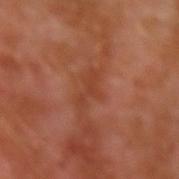Q: Is there a histopathology result?
A: imaged on a skin check; not biopsied
Q: Who is the patient?
A: male, aged 28–32
Q: How was the tile lit?
A: cross-polarized illumination
Q: What kind of image is this?
A: ~15 mm crop, total-body skin-cancer survey
Q: Where on the body is the lesion?
A: the left upper arm